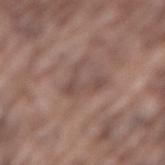| field | value |
|---|---|
| automated metrics | a footprint of about 7 mm², a shape eccentricity near 0.75, and a symmetry-axis asymmetry near 0.8; an average lesion color of about L≈48 a*≈17 b*≈22 (CIELAB), about 7 CIELAB-L* units darker than the surrounding skin, and a normalized border contrast of about 5.5; border irregularity of about 10 on a 0–10 scale and radial color variation of about 0.5; an automated nevus-likeness rating near 0 out of 100 |
| site | the lower back |
| subject | male, approximately 75 years of age |
| diameter | ~4.5 mm (longest diameter) |
| illumination | white-light |
| imaging modality | total-body-photography crop, ~15 mm field of view |